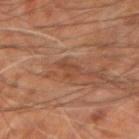workup = total-body-photography surveillance lesion; no biopsy
image = 15 mm crop, total-body photography
subject = male, approximately 60 years of age
site = the left arm